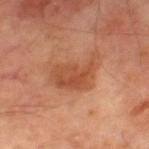notes — imaged on a skin check; not biopsied | anatomic site — the left thigh | patient — male, aged approximately 70 | tile lighting — cross-polarized | imaging modality — total-body-photography crop, ~15 mm field of view.